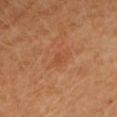Q: Was a biopsy performed?
A: no biopsy performed (imaged during a skin exam)
Q: How was the tile lit?
A: cross-polarized illumination
Q: What is the imaging modality?
A: total-body-photography crop, ~15 mm field of view
Q: Patient demographics?
A: female, approximately 65 years of age
Q: Where on the body is the lesion?
A: the head or neck
Q: Lesion size?
A: ≈1.5 mm